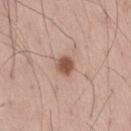| key | value |
|---|---|
| workup | imaged on a skin check; not biopsied |